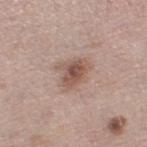Captured during whole-body skin photography for melanoma surveillance; the lesion was not biopsied.
Automated tile analysis of the lesion measured an area of roughly 11 mm², an eccentricity of roughly 0.65, and a symmetry-axis asymmetry near 0.35. And it measured an average lesion color of about L≈55 a*≈18 b*≈25 (CIELAB), a lesion–skin lightness drop of about 10, and a lesion-to-skin contrast of about 7 (normalized; higher = more distinct). The software also gave a nevus-likeness score of about 65/100 and lesion-presence confidence of about 100/100.
On the left thigh.
A female patient, aged 63–67.
Cropped from a total-body skin-imaging series; the visible field is about 15 mm.
Imaged with white-light lighting.
About 4 mm across.